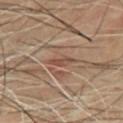No biopsy was performed on this lesion — it was imaged during a full skin examination and was not determined to be concerning. Cropped from a whole-body photographic skin survey; the tile spans about 15 mm. The recorded lesion diameter is about 3 mm. A male patient aged 58 to 62. From the chest. The lesion-visualizer software estimated an average lesion color of about L≈46 a*≈20 b*≈26 (CIELAB) and roughly 8 lightness units darker than nearby skin. Captured under cross-polarized illumination.